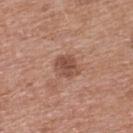subject = female, aged around 40 | location = the upper back | lesion diameter = about 3 mm | illumination = white-light illumination | image = total-body-photography crop, ~15 mm field of view | automated metrics = a mean CIELAB color near L≈50 a*≈21 b*≈28, a lesion–skin lightness drop of about 10, and a normalized border contrast of about 7.5; a lesion-detection confidence of about 100/100.